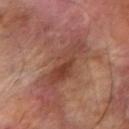Q: Was a biopsy performed?
A: no biopsy performed (imaged during a skin exam)
Q: How was this image acquired?
A: total-body-photography crop, ~15 mm field of view
Q: What are the patient's age and sex?
A: male, in their mid-60s
Q: What is the lesion's diameter?
A: ≈7 mm
Q: Automated lesion metrics?
A: a lesion color around L≈41 a*≈22 b*≈26 in CIELAB and roughly 10 lightness units darker than nearby skin; border irregularity of about 5 on a 0–10 scale and a within-lesion color-variation index near 6/10; a classifier nevus-likeness of about 0/100 and a lesion-detection confidence of about 90/100
Q: What is the anatomic site?
A: the arm
Q: What lighting was used for the tile?
A: cross-polarized